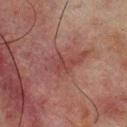Impression:
Recorded during total-body skin imaging; not selected for excision or biopsy.
Background:
A region of skin cropped from a whole-body photographic capture, roughly 15 mm wide. The lesion is on the back. A male patient in their mid- to late 60s. The lesion-visualizer software estimated a footprint of about 9.5 mm² and an outline eccentricity of about 0.9 (0 = round, 1 = elongated). And it measured an automated nevus-likeness rating near 0 out of 100 and a lesion-detection confidence of about 100/100. About 5.5 mm across.